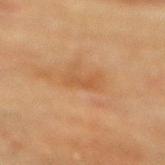Imaged during a routine full-body skin examination; the lesion was not biopsied and no histopathology is available. Captured under cross-polarized illumination. On the mid back. A 15 mm close-up tile from a total-body photography series done for melanoma screening. The total-body-photography lesion software estimated a lesion area of about 2 mm² and an eccentricity of roughly 0.95. The analysis additionally found a mean CIELAB color near L≈46 a*≈21 b*≈35. About 2.5 mm across. A female subject, about 80 years old.